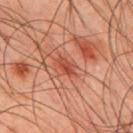Assessment:
Part of a total-body skin-imaging series; this lesion was reviewed on a skin check and was not flagged for biopsy.
Clinical summary:
The tile uses cross-polarized illumination. This image is a 15 mm lesion crop taken from a total-body photograph. A male patient about 45 years old. Located on the mid back. Approximately 3 mm at its widest.